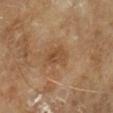This lesion was catalogued during total-body skin photography and was not selected for biopsy. An algorithmic analysis of the crop reported a border-irregularity index near 3/10, a color-variation rating of about 3.5/10, and radial color variation of about 1.5. And it measured a lesion-detection confidence of about 100/100. Cropped from a whole-body photographic skin survey; the tile spans about 15 mm. The lesion's longest dimension is about 3.5 mm. On the right lower leg. The patient is a male roughly 65 years of age.